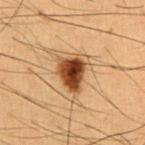Findings:
• patient — male, approximately 50 years of age
• tile lighting — cross-polarized illumination
• image source — 15 mm crop, total-body photography
• anatomic site — the abdomen
• lesion size — about 4.5 mm
• automated metrics — an eccentricity of roughly 0.65 and a shape-asymmetry score of about 0.4 (0 = symmetric); border irregularity of about 3.5 on a 0–10 scale, internal color variation of about 7.5 on a 0–10 scale, and a peripheral color-asymmetry measure near 2; a nevus-likeness score of about 100/100